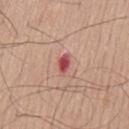<tbp_lesion>
<site>mid back</site>
<lesion_size>
  <long_diameter_mm_approx>3.0</long_diameter_mm_approx>
</lesion_size>
<image>
  <source>total-body photography crop</source>
  <field_of_view_mm>15</field_of_view_mm>
</image>
<patient>
  <sex>male</sex>
  <age_approx>70</age_approx>
</patient>
</tbp_lesion>